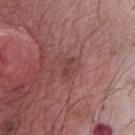biopsy_status: not biopsied; imaged during a skin examination
image:
  source: total-body photography crop
  field_of_view_mm: 15
patient:
  sex: male
  age_approx: 65
automated_metrics:
  area_mm2_approx: 3.5
  eccentricity: 0.85
  shape_asymmetry: 0.3
  cielab_L: 42
  cielab_a: 23
  cielab_b: 23
  vs_skin_darker_L: 6.0
  peripheral_color_asymmetry: 0.0
  lesion_detection_confidence_0_100: 100
lesion_size:
  long_diameter_mm_approx: 2.5
lighting: white-light
site: chest